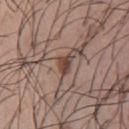Part of a total-body skin-imaging series; this lesion was reviewed on a skin check and was not flagged for biopsy. The lesion is located on the front of the torso. Imaged with white-light lighting. The subject is a male about 30 years old. A close-up tile cropped from a whole-body skin photograph, about 15 mm across. The lesion's longest dimension is about 2.5 mm.